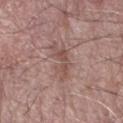notes: total-body-photography surveillance lesion; no biopsy
tile lighting: white-light illumination
acquisition: ~15 mm crop, total-body skin-cancer survey
anatomic site: the right forearm
TBP lesion metrics: a lesion color around L≈51 a*≈19 b*≈22 in CIELAB and roughly 8 lightness units darker than nearby skin
size: ≈5 mm
patient: male, about 70 years old